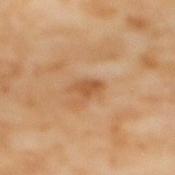Imaged during a routine full-body skin examination; the lesion was not biopsied and no histopathology is available. Imaged with cross-polarized lighting. A female subject in their mid-50s. From the mid back. A 15 mm close-up tile from a total-body photography series done for melanoma screening.